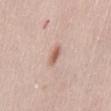| field | value |
|---|---|
| biopsy status | catalogued during a skin exam; not biopsied |
| illumination | white-light illumination |
| patient | female, about 30 years old |
| anatomic site | the abdomen |
| size | ~3 mm (longest diameter) |
| acquisition | 15 mm crop, total-body photography |